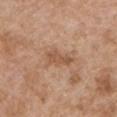The lesion was photographed on a routine skin check and not biopsied; there is no pathology result.